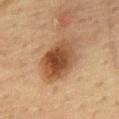The lesion was tiled from a total-body skin photograph and was not biopsied.
The lesion is located on the upper back.
This is a cross-polarized tile.
The recorded lesion diameter is about 5.5 mm.
A lesion tile, about 15 mm wide, cut from a 3D total-body photograph.
A male patient, aged approximately 65.